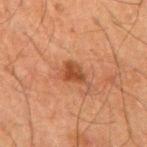Part of a total-body skin-imaging series; this lesion was reviewed on a skin check and was not flagged for biopsy. Captured under cross-polarized illumination. An algorithmic analysis of the crop reported roughly 10 lightness units darker than nearby skin and a lesion-to-skin contrast of about 8 (normalized; higher = more distinct). The analysis additionally found a peripheral color-asymmetry measure near 1. The patient is a male about 65 years old. This image is a 15 mm lesion crop taken from a total-body photograph. The lesion is located on the right upper arm.